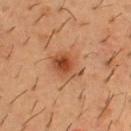biopsy status=catalogued during a skin exam; not biopsied | patient=male, about 55 years old | illumination=cross-polarized illumination | anatomic site=the chest | automated lesion analysis=peripheral color asymmetry of about 1.5 | size=~4 mm (longest diameter) | image source=~15 mm crop, total-body skin-cancer survey.